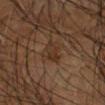workup: imaged on a skin check; not biopsied
patient: male, roughly 55 years of age
body site: the right forearm
lighting: cross-polarized illumination
image-analysis metrics: a mean CIELAB color near L≈29 a*≈15 b*≈25 and a normalized border contrast of about 6
lesion diameter: ≈3 mm
image source: 15 mm crop, total-body photography